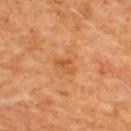Clinical summary:
Located on the upper back. The subject is a female aged approximately 50. Cropped from a whole-body photographic skin survey; the tile spans about 15 mm. The total-body-photography lesion software estimated a lesion color around L≈55 a*≈27 b*≈43 in CIELAB, a lesion–skin lightness drop of about 7, and a normalized lesion–skin contrast near 5.5. The analysis additionally found an automated nevus-likeness rating near 0 out of 100. About 2.5 mm across.